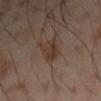<tbp_lesion>
<biopsy_status>not biopsied; imaged during a skin examination</biopsy_status>
<lighting>cross-polarized</lighting>
<site>left arm</site>
<patient>
  <sex>male</sex>
  <age_approx>50</age_approx>
</patient>
<image>
  <source>total-body photography crop</source>
  <field_of_view_mm>15</field_of_view_mm>
</image>
<automated_metrics>
  <area_mm2_approx>5.5</area_mm2_approx>
  <eccentricity>0.75</eccentricity>
  <shape_asymmetry>0.3</shape_asymmetry>
  <cielab_L>34</cielab_L>
  <cielab_a>15</cielab_a>
  <cielab_b>23</cielab_b>
  <vs_skin_contrast_norm>7.5</vs_skin_contrast_norm>
  <color_variation_0_10>3.0</color_variation_0_10>
  <peripheral_color_asymmetry>1.0</peripheral_color_asymmetry>
</automated_metrics>
</tbp_lesion>